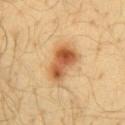Case summary:
– follow-up · no biopsy performed (imaged during a skin exam)
– patient · male, in their 40s
– image · total-body-photography crop, ~15 mm field of view
– body site · the chest